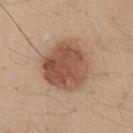The total-body-photography lesion software estimated a footprint of about 24 mm² and two-axis asymmetry of about 0.15. And it measured an average lesion color of about L≈52 a*≈22 b*≈29 (CIELAB), about 13 CIELAB-L* units darker than the surrounding skin, and a normalized lesion–skin contrast near 9. And it measured a classifier nevus-likeness of about 100/100 and a lesion-detection confidence of about 100/100.
The lesion's longest dimension is about 6 mm.
Cropped from a whole-body photographic skin survey; the tile spans about 15 mm.
This is a white-light tile.
The lesion is located on the abdomen.
The subject is a male aged 33–37.